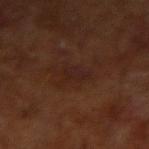biopsy status: no biopsy performed (imaged during a skin exam); lighting: cross-polarized illumination; TBP lesion metrics: a nevus-likeness score of about 0/100; subject: male, about 65 years old; anatomic site: the right forearm; image: total-body-photography crop, ~15 mm field of view; size: ≈3 mm.